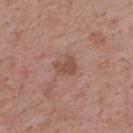{
  "biopsy_status": "not biopsied; imaged during a skin examination",
  "automated_metrics": {
    "area_mm2_approx": 4.0,
    "eccentricity": 0.65,
    "shape_asymmetry": 0.4,
    "nevus_likeness_0_100": 0
  },
  "patient": {
    "sex": "male",
    "age_approx": 70
  },
  "site": "back",
  "lesion_size": {
    "long_diameter_mm_approx": 2.5
  },
  "image": {
    "source": "total-body photography crop",
    "field_of_view_mm": 15
  },
  "lighting": "white-light"
}